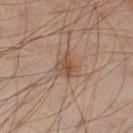illumination = cross-polarized illumination; image source = ~15 mm crop, total-body skin-cancer survey; patient = male, in their mid- to late 50s; body site = the leg.